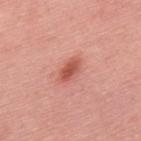Clinical impression:
Part of a total-body skin-imaging series; this lesion was reviewed on a skin check and was not flagged for biopsy.
Clinical summary:
Approximately 3.5 mm at its widest. A 15 mm close-up tile from a total-body photography series done for melanoma screening. The tile uses white-light illumination. A male subject, in their mid-50s. On the back. Automated image analysis of the tile measured a symmetry-axis asymmetry near 0.15. The software also gave a lesion color around L≈56 a*≈31 b*≈30 in CIELAB, about 12 CIELAB-L* units darker than the surrounding skin, and a normalized border contrast of about 8.